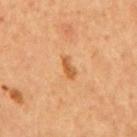This lesion was catalogued during total-body skin photography and was not selected for biopsy. The lesion is on the back. The total-body-photography lesion software estimated an area of roughly 2.5 mm² and a shape eccentricity near 0.9. It also reported a classifier nevus-likeness of about 50/100 and a detector confidence of about 100 out of 100 that the crop contains a lesion. Cropped from a whole-body photographic skin survey; the tile spans about 15 mm. Approximately 3 mm at its widest. A male subject in their mid-50s. Captured under cross-polarized illumination.